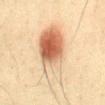notes=imaged on a skin check; not biopsied
site=the abdomen
illumination=cross-polarized
image=~15 mm tile from a whole-body skin photo
patient=male, aged 38 to 42